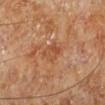Assessment: Captured during whole-body skin photography for melanoma surveillance; the lesion was not biopsied.